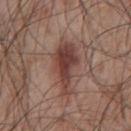The tile uses white-light illumination.
Automated tile analysis of the lesion measured an average lesion color of about L≈41 a*≈21 b*≈23 (CIELAB) and a normalized border contrast of about 9.
Cropped from a total-body skin-imaging series; the visible field is about 15 mm.
Located on the chest.
A male patient, in their mid- to late 50s.
Measured at roughly 6.5 mm in maximum diameter.